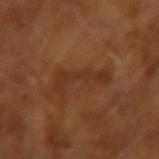| field | value |
|---|---|
| notes | catalogued during a skin exam; not biopsied |
| acquisition | total-body-photography crop, ~15 mm field of view |
| tile lighting | cross-polarized illumination |
| subject | male, approximately 65 years of age |